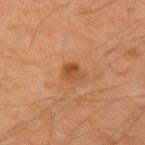Q: Was a biopsy performed?
A: total-body-photography surveillance lesion; no biopsy
Q: What kind of image is this?
A: 15 mm crop, total-body photography
Q: Lesion location?
A: the left upper arm
Q: What are the patient's age and sex?
A: male, about 45 years old